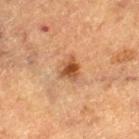| field | value |
|---|---|
| image | ~15 mm tile from a whole-body skin photo |
| patient | male, about 75 years old |
| body site | the left thigh |